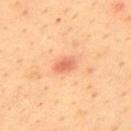Assessment:
No biopsy was performed on this lesion — it was imaged during a full skin examination and was not determined to be concerning.
Context:
Automated tile analysis of the lesion measured a footprint of about 4 mm², a shape eccentricity near 0.75, and a shape-asymmetry score of about 0.15 (0 = symmetric). The software also gave a mean CIELAB color near L≈61 a*≈28 b*≈35 and a lesion–skin lightness drop of about 10. The patient is a male about 35 years old. About 2.5 mm across. A 15 mm close-up tile from a total-body photography series done for melanoma screening. From the upper back. Imaged with cross-polarized lighting.